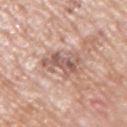Assessment: Recorded during total-body skin imaging; not selected for excision or biopsy. Acquisition and patient details: Automated image analysis of the tile measured a shape eccentricity near 0.85 and a shape-asymmetry score of about 0.4 (0 = symmetric). And it measured about 11 CIELAB-L* units darker than the surrounding skin. The analysis additionally found a color-variation rating of about 6/10. The analysis additionally found a nevus-likeness score of about 0/100 and a lesion-detection confidence of about 80/100. A 15 mm close-up extracted from a 3D total-body photography capture. Measured at roughly 5 mm in maximum diameter. From the right upper arm. A male subject about 70 years old.